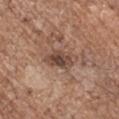Assessment: Recorded during total-body skin imaging; not selected for excision or biopsy. Context: Imaged with white-light lighting. Longest diameter approximately 4 mm. This image is a 15 mm lesion crop taken from a total-body photograph. Located on the left upper arm. The patient is a male aged 68–72.